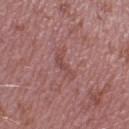Assessment: Captured during whole-body skin photography for melanoma surveillance; the lesion was not biopsied. Background: The lesion is located on the right lower leg. The recorded lesion diameter is about 3.5 mm. A region of skin cropped from a whole-body photographic capture, roughly 15 mm wide. A male patient, aged approximately 40.